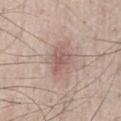Q: Automated lesion metrics?
A: a footprint of about 6.5 mm² and a shape-asymmetry score of about 0.25 (0 = symmetric); an average lesion color of about L≈57 a*≈19 b*≈22 (CIELAB) and a normalized border contrast of about 6; a border-irregularity index near 2.5/10, a within-lesion color-variation index near 3.5/10, and peripheral color asymmetry of about 1
Q: Patient demographics?
A: male, in their 50s
Q: What is the imaging modality?
A: 15 mm crop, total-body photography
Q: What is the anatomic site?
A: the chest